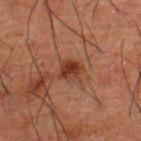biopsy status — catalogued during a skin exam; not biopsied
location — the upper back
lesion diameter — ~3 mm (longest diameter)
imaging modality — total-body-photography crop, ~15 mm field of view
subject — male, approximately 50 years of age